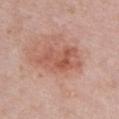Assessment:
Recorded during total-body skin imaging; not selected for excision or biopsy.
Acquisition and patient details:
A female patient, aged 48–52. A 15 mm close-up tile from a total-body photography series done for melanoma screening. From the chest.